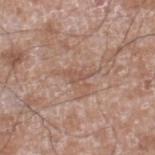workup — no biopsy performed (imaged during a skin exam) | location — the right lower leg | patient — male, aged around 60 | illumination — white-light | lesion size — ≈3 mm | image source — total-body-photography crop, ~15 mm field of view.